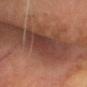follow-up = catalogued during a skin exam; not biopsied | subject = male, aged around 65 | anatomic site = the head or neck | image source = ~15 mm tile from a whole-body skin photo.